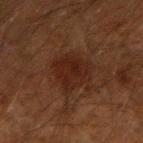Recorded during total-body skin imaging; not selected for excision or biopsy. A male patient aged around 50. A 15 mm crop from a total-body photograph taken for skin-cancer surveillance. On the right forearm. Longest diameter approximately 4 mm.